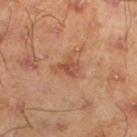Clinical impression:
Imaged during a routine full-body skin examination; the lesion was not biopsied and no histopathology is available.
Image and clinical context:
An algorithmic analysis of the crop reported an area of roughly 5 mm². The software also gave border irregularity of about 4 on a 0–10 scale, internal color variation of about 5.5 on a 0–10 scale, and radial color variation of about 2. Imaged with cross-polarized lighting. A lesion tile, about 15 mm wide, cut from a 3D total-body photograph. On the left lower leg. Measured at roughly 3 mm in maximum diameter. A male subject, approximately 45 years of age.